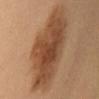| feature | finding |
|---|---|
| illumination | cross-polarized |
| location | the left upper arm |
| automated lesion analysis | a lesion area of about 44 mm², an eccentricity of roughly 0.85, and a shape-asymmetry score of about 0.2 (0 = symmetric); a mean CIELAB color near L≈47 a*≈20 b*≈33, roughly 12 lightness units darker than nearby skin, and a lesion-to-skin contrast of about 8.5 (normalized; higher = more distinct); a within-lesion color-variation index near 5.5/10 and radial color variation of about 1.5 |
| subject | female, aged 38–42 |
| image source | total-body-photography crop, ~15 mm field of view |
| diameter | ~10.5 mm (longest diameter) |